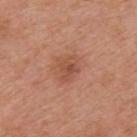No biopsy was performed on this lesion — it was imaged during a full skin examination and was not determined to be concerning.
A male patient, about 70 years old.
On the mid back.
Approximately 3 mm at its widest.
Imaged with white-light lighting.
The lesion-visualizer software estimated a footprint of about 5 mm², a shape eccentricity near 0.65, and a shape-asymmetry score of about 0.3 (0 = symmetric). The analysis additionally found an average lesion color of about L≈51 a*≈25 b*≈31 (CIELAB) and a normalized lesion–skin contrast near 6. The analysis additionally found a color-variation rating of about 3/10 and a peripheral color-asymmetry measure near 1.
A 15 mm crop from a total-body photograph taken for skin-cancer surveillance.